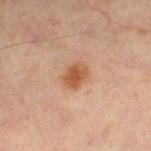Q: What lighting was used for the tile?
A: cross-polarized illumination
Q: How was this image acquired?
A: total-body-photography crop, ~15 mm field of view
Q: Where on the body is the lesion?
A: the left lower leg
Q: Automated lesion metrics?
A: a mean CIELAB color near L≈52 a*≈21 b*≈33, roughly 10 lightness units darker than nearby skin, and a normalized border contrast of about 8; border irregularity of about 1.5 on a 0–10 scale, internal color variation of about 3.5 on a 0–10 scale, and peripheral color asymmetry of about 1
Q: How large is the lesion?
A: ~3 mm (longest diameter)
Q: Patient demographics?
A: male, aged approximately 60